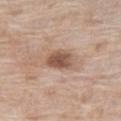{"biopsy_status": "not biopsied; imaged during a skin examination", "automated_metrics": {"eccentricity": 0.8, "shape_asymmetry": 0.25, "cielab_L": 54, "cielab_a": 19, "cielab_b": 28, "vs_skin_contrast_norm": 9.0}, "lighting": "white-light", "site": "right thigh", "lesion_size": {"long_diameter_mm_approx": 4.0}, "patient": {"sex": "female", "age_approx": 75}, "image": {"source": "total-body photography crop", "field_of_view_mm": 15}}